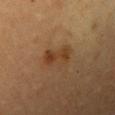<lesion>
  <biopsy_status>not biopsied; imaged during a skin examination</biopsy_status>
  <lesion_size>
    <long_diameter_mm_approx>4.0</long_diameter_mm_approx>
  </lesion_size>
  <patient>
    <sex>female</sex>
    <age_approx>60</age_approx>
  </patient>
  <image>
    <source>total-body photography crop</source>
    <field_of_view_mm>15</field_of_view_mm>
  </image>
  <lighting>cross-polarized</lighting>
  <site>left upper arm</site>
</lesion>